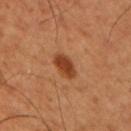{"biopsy_status": "not biopsied; imaged during a skin examination", "lighting": "cross-polarized", "site": "right upper arm", "image": {"source": "total-body photography crop", "field_of_view_mm": 15}, "patient": {"sex": "male", "age_approx": 50}, "lesion_size": {"long_diameter_mm_approx": 3.5}}